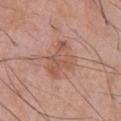notes: imaged on a skin check; not biopsied | size: ~4.5 mm (longest diameter) | tile lighting: white-light illumination | patient: male, roughly 60 years of age | location: the chest | image source: ~15 mm crop, total-body skin-cancer survey.